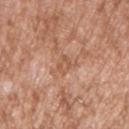• automated lesion analysis: an area of roughly 3.5 mm², an outline eccentricity of about 0.75 (0 = round, 1 = elongated), and a symmetry-axis asymmetry near 0.35; roughly 7 lightness units darker than nearby skin and a lesion-to-skin contrast of about 5.5 (normalized; higher = more distinct); a border-irregularity rating of about 3/10, internal color variation of about 2 on a 0–10 scale, and peripheral color asymmetry of about 1; a classifier nevus-likeness of about 0/100 and a lesion-detection confidence of about 100/100
• acquisition: total-body-photography crop, ~15 mm field of view
• site: the right upper arm
• subject: male, aged approximately 45